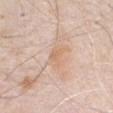  site: front of the torso
  image:
    source: total-body photography crop
    field_of_view_mm: 15
  patient:
    sex: male
    age_approx: 80